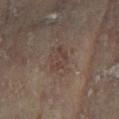workup = total-body-photography surveillance lesion; no biopsy
tile lighting = cross-polarized illumination
subject = female, aged 73–77
image = ~15 mm tile from a whole-body skin photo
site = the leg
lesion diameter = ~3.5 mm (longest diameter)